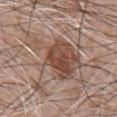Q: Was a biopsy performed?
A: catalogued during a skin exam; not biopsied
Q: How was this image acquired?
A: ~15 mm tile from a whole-body skin photo
Q: Illumination type?
A: white-light
Q: Where on the body is the lesion?
A: the chest
Q: Patient demographics?
A: male, aged 58–62
Q: What did automated image analysis measure?
A: an area of roughly 28 mm², an eccentricity of roughly 0.8, and a shape-asymmetry score of about 0.45 (0 = symmetric); a lesion color around L≈50 a*≈18 b*≈25 in CIELAB, a lesion–skin lightness drop of about 10, and a lesion-to-skin contrast of about 7.5 (normalized; higher = more distinct); border irregularity of about 7 on a 0–10 scale, internal color variation of about 6 on a 0–10 scale, and peripheral color asymmetry of about 1.5; a nevus-likeness score of about 50/100
Q: What is the lesion's diameter?
A: ≈9.5 mm